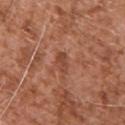Part of a total-body skin-imaging series; this lesion was reviewed on a skin check and was not flagged for biopsy.
From the back.
A lesion tile, about 15 mm wide, cut from a 3D total-body photograph.
A male patient about 75 years old.
An algorithmic analysis of the crop reported a lesion area of about 3.5 mm², an eccentricity of roughly 0.75, and a shape-asymmetry score of about 0.25 (0 = symmetric).